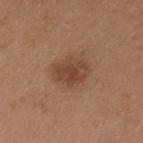Impression:
Captured during whole-body skin photography for melanoma surveillance; the lesion was not biopsied.
Background:
Longest diameter approximately 4 mm. A 15 mm close-up tile from a total-body photography series done for melanoma screening. The subject is a female aged 38–42. The lesion is located on the right upper arm. This is a white-light tile.